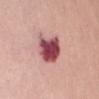  biopsy_status: not biopsied; imaged during a skin examination
  image:
    source: total-body photography crop
    field_of_view_mm: 15
  patient:
    sex: female
    age_approx: 65
  site: abdomen
  lighting: white-light
  lesion_size:
    long_diameter_mm_approx: 4.0